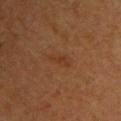This lesion was catalogued during total-body skin photography and was not selected for biopsy. The recorded lesion diameter is about 3 mm. A female subject aged around 50. Located on the left upper arm. A close-up tile cropped from a whole-body skin photograph, about 15 mm across. Captured under cross-polarized illumination.